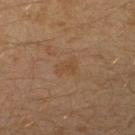Captured during whole-body skin photography for melanoma surveillance; the lesion was not biopsied. The tile uses cross-polarized illumination. A lesion tile, about 15 mm wide, cut from a 3D total-body photograph. The lesion is on the leg. The lesion's longest dimension is about 2.5 mm. A male patient, aged 28 to 32.